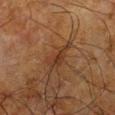follow-up: catalogued during a skin exam; not biopsied.